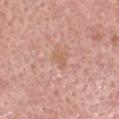Q: Is there a histopathology result?
A: imaged on a skin check; not biopsied
Q: What is the lesion's diameter?
A: ≈2 mm
Q: How was this image acquired?
A: 15 mm crop, total-body photography
Q: Who is the patient?
A: male, aged around 60
Q: Illumination type?
A: white-light
Q: Lesion location?
A: the head or neck
Q: What did automated image analysis measure?
A: an area of roughly 2 mm²; a border-irregularity index near 3.5/10 and peripheral color asymmetry of about 0